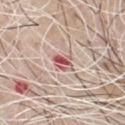Assessment:
Recorded during total-body skin imaging; not selected for excision or biopsy.
Background:
Imaged with white-light lighting. Located on the chest. A male subject aged 68–72. A 15 mm close-up tile from a total-body photography series done for melanoma screening. The total-body-photography lesion software estimated a lesion color around L≈57 a*≈27 b*≈23 in CIELAB and a lesion–skin lightness drop of about 14. The software also gave a color-variation rating of about 8/10 and peripheral color asymmetry of about 3. The software also gave an automated nevus-likeness rating near 0 out of 100 and a detector confidence of about 100 out of 100 that the crop contains a lesion. Measured at roughly 2.5 mm in maximum diameter.